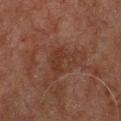No biopsy was performed on this lesion — it was imaged during a full skin examination and was not determined to be concerning. The tile uses cross-polarized illumination. Cropped from a whole-body photographic skin survey; the tile spans about 15 mm. From the chest. A male patient aged approximately 60.